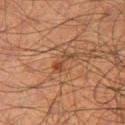notes = total-body-photography surveillance lesion; no biopsy
anatomic site = the right thigh
automated metrics = a lesion area of about 3.5 mm²
diameter = about 3.5 mm
subject = male, aged approximately 50
lighting = cross-polarized
imaging modality = ~15 mm tile from a whole-body skin photo